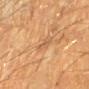Findings:
* workup — total-body-photography surveillance lesion; no biopsy
* subject — male, aged around 60
* size — ≈3 mm
* tile lighting — cross-polarized
* site — the left forearm
* acquisition — total-body-photography crop, ~15 mm field of view